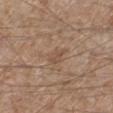Recorded during total-body skin imaging; not selected for excision or biopsy.
This is a white-light tile.
A 15 mm crop from a total-body photograph taken for skin-cancer surveillance.
From the left lower leg.
A male subject, aged 63 to 67.
About 2.5 mm across.